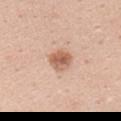| feature | finding |
|---|---|
| biopsy status | total-body-photography surveillance lesion; no biopsy |
| body site | the right upper arm |
| diameter | about 3 mm |
| imaging modality | total-body-photography crop, ~15 mm field of view |
| patient | female, aged approximately 25 |